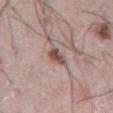Imaged during a routine full-body skin examination; the lesion was not biopsied and no histopathology is available.
Automated tile analysis of the lesion measured a nevus-likeness score of about 85/100 and lesion-presence confidence of about 100/100.
A male subject roughly 75 years of age.
The lesion is on the abdomen.
Cropped from a whole-body photographic skin survey; the tile spans about 15 mm.
This is a white-light tile.